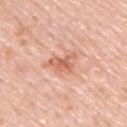| key | value |
|---|---|
| biopsy status | total-body-photography surveillance lesion; no biopsy |